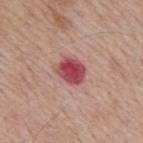  biopsy_status: not biopsied; imaged during a skin examination
  image:
    source: total-body photography crop
    field_of_view_mm: 15
  lighting: white-light
  site: mid back
  lesion_size:
    long_diameter_mm_approx: 3.5
  automated_metrics:
    cielab_L: 47
    cielab_a: 35
    cielab_b: 21
    vs_skin_darker_L: 16.0
    vs_skin_contrast_norm: 11.5
  patient:
    sex: male
    age_approx: 60